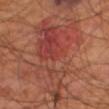The total-body-photography lesion software estimated a lesion color around L≈41 a*≈27 b*≈28 in CIELAB and a lesion–skin lightness drop of about 7. And it measured a border-irregularity rating of about 7.5/10 and a peripheral color-asymmetry measure near 2.5. The software also gave an automated nevus-likeness rating near 85 out of 100 and a detector confidence of about 100 out of 100 that the crop contains a lesion. The lesion's longest dimension is about 12.5 mm. Captured under cross-polarized illumination. A male patient, in their 70s. A lesion tile, about 15 mm wide, cut from a 3D total-body photograph. On the leg.